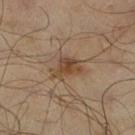| feature | finding |
|---|---|
| biopsy status | total-body-photography surveillance lesion; no biopsy |
| illumination | cross-polarized illumination |
| image | ~15 mm crop, total-body skin-cancer survey |
| body site | the right lower leg |
| subject | male, about 65 years old |